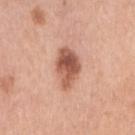biopsy_status: not biopsied; imaged during a skin examination
lighting: white-light
image:
  source: total-body photography crop
  field_of_view_mm: 15
patient:
  sex: female
  age_approx: 60
automated_metrics:
  cielab_L: 56
  cielab_a: 24
  cielab_b: 30
  vs_skin_contrast_norm: 9.5
  nevus_likeness_0_100: 80
  lesion_detection_confidence_0_100: 100
site: right upper arm
lesion_size:
  long_diameter_mm_approx: 5.0